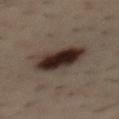The lesion was photographed on a routine skin check and not biopsied; there is no pathology result. A lesion tile, about 15 mm wide, cut from a 3D total-body photograph. A male subject, aged 38–42. Located on the mid back.